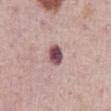Clinical impression: The lesion was photographed on a routine skin check and not biopsied; there is no pathology result. Context: On the abdomen. A close-up tile cropped from a whole-body skin photograph, about 15 mm across. The patient is a male aged around 75.